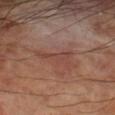workup — imaged on a skin check; not biopsied
patient — male, approximately 70 years of age
lesion size — ≈4.5 mm
site — the left forearm
imaging modality — 15 mm crop, total-body photography
lighting — cross-polarized illumination
automated metrics — a border-irregularity rating of about 7.5/10; an automated nevus-likeness rating near 0 out of 100